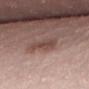follow-up: catalogued during a skin exam; not biopsied | imaging modality: ~15 mm tile from a whole-body skin photo | diameter: ~3 mm (longest diameter) | patient: female, aged approximately 30 | lighting: white-light | anatomic site: the back.